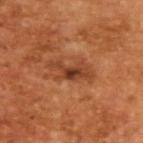This lesion was catalogued during total-body skin photography and was not selected for biopsy. A roughly 15 mm field-of-view crop from a total-body skin photograph. A male subject, aged 63–67.